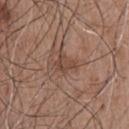Findings:
• workup: imaged on a skin check; not biopsied
• illumination: white-light illumination
• subject: male, about 50 years old
• lesion size: about 3 mm
• body site: the upper back
• image source: ~15 mm crop, total-body skin-cancer survey